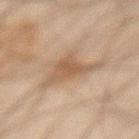{
  "biopsy_status": "not biopsied; imaged during a skin examination",
  "patient": {
    "sex": "male",
    "age_approx": 45
  },
  "lesion_size": {
    "long_diameter_mm_approx": 5.0
  },
  "image": {
    "source": "total-body photography crop",
    "field_of_view_mm": 15
  },
  "site": "abdomen",
  "automated_metrics": {
    "cielab_L": 45,
    "cielab_a": 14,
    "cielab_b": 26,
    "vs_skin_contrast_norm": 6.5,
    "nevus_likeness_0_100": 0,
    "lesion_detection_confidence_0_100": 100
  },
  "lighting": "cross-polarized"
}